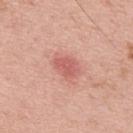workup: imaged on a skin check; not biopsied | body site: the back | lesion diameter: ≈3 mm | subject: male, aged 38–42 | image source: total-body-photography crop, ~15 mm field of view.